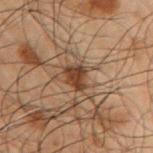This lesion was catalogued during total-body skin photography and was not selected for biopsy.
The subject is a male roughly 50 years of age.
From the arm.
A close-up tile cropped from a whole-body skin photograph, about 15 mm across.
Captured under cross-polarized illumination.
An algorithmic analysis of the crop reported a lesion area of about 5 mm² and a symmetry-axis asymmetry near 0.35. It also reported an automated nevus-likeness rating near 85 out of 100 and lesion-presence confidence of about 100/100.
The lesion's longest dimension is about 3 mm.